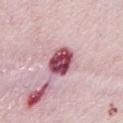notes = imaged on a skin check; not biopsied
patient = female, aged around 65
image-analysis metrics = an average lesion color of about L≈50 a*≈32 b*≈14 (CIELAB), roughly 23 lightness units darker than nearby skin, and a normalized lesion–skin contrast near 14; border irregularity of about 1.5 on a 0–10 scale and a color-variation rating of about 7.5/10
image source = total-body-photography crop, ~15 mm field of view
size = ≈4 mm
tile lighting = white-light
body site = the chest